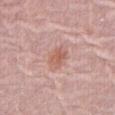Recorded during total-body skin imaging; not selected for excision or biopsy.
The tile uses white-light illumination.
A female patient aged 63 to 67.
The lesion's longest dimension is about 3.5 mm.
From the abdomen.
A region of skin cropped from a whole-body photographic capture, roughly 15 mm wide.